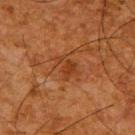The subject is a male approximately 65 years of age.
The lesion-visualizer software estimated a shape eccentricity near 0.75 and a shape-asymmetry score of about 0.4 (0 = symmetric). And it measured a lesion-to-skin contrast of about 6 (normalized; higher = more distinct). The software also gave border irregularity of about 4.5 on a 0–10 scale and a color-variation rating of about 1.5/10.
Longest diameter approximately 3 mm.
A roughly 15 mm field-of-view crop from a total-body skin photograph.
Located on the upper back.
This is a cross-polarized tile.